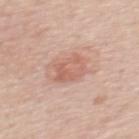workup = no biopsy performed (imaged during a skin exam)
acquisition = ~15 mm crop, total-body skin-cancer survey
diameter = about 3.5 mm
subject = female, in their mid- to late 40s
body site = the upper back
illumination = white-light